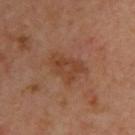The subject is a male about 65 years old. The tile uses cross-polarized illumination. Located on the upper back. Cropped from a total-body skin-imaging series; the visible field is about 15 mm.